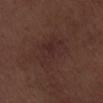Notes:
– follow-up · imaged on a skin check; not biopsied
– subject · male, aged around 70
– image · 15 mm crop, total-body photography
– location · the right lower leg